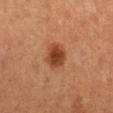About 3 mm across. On the right lower leg. The lesion-visualizer software estimated a lesion area of about 7 mm², a shape eccentricity near 0.35, and a symmetry-axis asymmetry near 0.15. It also reported a nevus-likeness score of about 100/100 and a lesion-detection confidence of about 100/100. Cropped from a whole-body photographic skin survey; the tile spans about 15 mm. The patient is a female aged approximately 55.